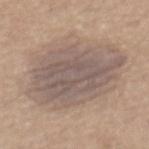follow-up=imaged on a skin check; not biopsied | patient=male, roughly 65 years of age | body site=the back | lighting=white-light illumination | lesion diameter=≈9.5 mm | imaging modality=total-body-photography crop, ~15 mm field of view.